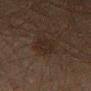Q: Was a biopsy performed?
A: total-body-photography surveillance lesion; no biopsy
Q: Automated lesion metrics?
A: a footprint of about 7.5 mm², an outline eccentricity of about 0.9 (0 = round, 1 = elongated), and two-axis asymmetry of about 0.2; a border-irregularity index near 2.5/10, a within-lesion color-variation index near 2/10, and radial color variation of about 0.5; a nevus-likeness score of about 0/100 and lesion-presence confidence of about 100/100
Q: Patient demographics?
A: male, approximately 60 years of age
Q: What is the imaging modality?
A: total-body-photography crop, ~15 mm field of view
Q: Lesion location?
A: the leg
Q: How large is the lesion?
A: about 4.5 mm
Q: What lighting was used for the tile?
A: cross-polarized